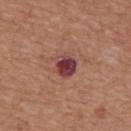Q: Was a biopsy performed?
A: catalogued during a skin exam; not biopsied
Q: Who is the patient?
A: male, approximately 65 years of age
Q: What is the anatomic site?
A: the mid back
Q: How was this image acquired?
A: 15 mm crop, total-body photography
Q: Lesion size?
A: ~3 mm (longest diameter)
Q: What lighting was used for the tile?
A: white-light illumination